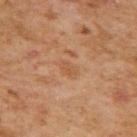The lesion was photographed on a routine skin check and not biopsied; there is no pathology result.
The lesion is located on the upper back.
Automated tile analysis of the lesion measured a classifier nevus-likeness of about 0/100 and a detector confidence of about 100 out of 100 that the crop contains a lesion.
Measured at roughly 2.5 mm in maximum diameter.
Cropped from a whole-body photographic skin survey; the tile spans about 15 mm.
A female patient, approximately 60 years of age.